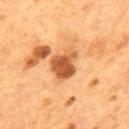Image and clinical context:
Measured at roughly 4 mm in maximum diameter. Cropped from a total-body skin-imaging series; the visible field is about 15 mm. A male patient about 55 years old. The total-body-photography lesion software estimated border irregularity of about 3 on a 0–10 scale, internal color variation of about 5 on a 0–10 scale, and a peripheral color-asymmetry measure near 1.5. This is a cross-polarized tile. The lesion is located on the mid back.